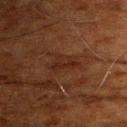Findings:
* follow-up — catalogued during a skin exam; not biopsied
* subject — male, roughly 80 years of age
* anatomic site — the head or neck
* diameter — ≈3.5 mm
* automated metrics — a footprint of about 4.5 mm², an outline eccentricity of about 0.85 (0 = round, 1 = elongated), and a symmetry-axis asymmetry near 0.55; a classifier nevus-likeness of about 0/100 and a detector confidence of about 95 out of 100 that the crop contains a lesion
* image source — ~15 mm crop, total-body skin-cancer survey